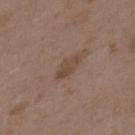Clinical impression:
This lesion was catalogued during total-body skin photography and was not selected for biopsy.
Background:
A female patient, about 35 years old. A close-up tile cropped from a whole-body skin photograph, about 15 mm across. On the upper back.